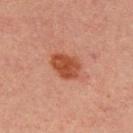Q: Was this lesion biopsied?
A: no biopsy performed (imaged during a skin exam)
Q: Patient demographics?
A: male, aged approximately 30
Q: What did automated image analysis measure?
A: an area of roughly 8.5 mm², an eccentricity of roughly 0.7, and a shape-asymmetry score of about 0.2 (0 = symmetric)
Q: How large is the lesion?
A: about 3.5 mm
Q: How was the tile lit?
A: cross-polarized illumination
Q: Lesion location?
A: the upper back
Q: What is the imaging modality?
A: total-body-photography crop, ~15 mm field of view